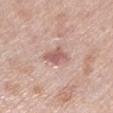<tbp_lesion>
  <biopsy_status>not biopsied; imaged during a skin examination</biopsy_status>
  <patient>
    <sex>female</sex>
    <age_approx>65</age_approx>
  </patient>
  <automated_metrics>
    <cielab_L>59</cielab_L>
    <cielab_a>22</cielab_a>
    <cielab_b>24</cielab_b>
    <border_irregularity_0_10>2.5</border_irregularity_0_10>
    <peripheral_color_asymmetry>1.0</peripheral_color_asymmetry>
    <nevus_likeness_0_100>5</nevus_likeness_0_100>
  </automated_metrics>
  <lesion_size>
    <long_diameter_mm_approx>3.0</long_diameter_mm_approx>
  </lesion_size>
  <site>left lower leg</site>
  <image>
    <source>total-body photography crop</source>
    <field_of_view_mm>15</field_of_view_mm>
  </image>
  <lighting>white-light</lighting>
</tbp_lesion>